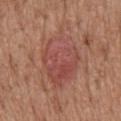Clinical impression: No biopsy was performed on this lesion — it was imaged during a full skin examination and was not determined to be concerning. Clinical summary: The lesion-visualizer software estimated an area of roughly 25 mm², an outline eccentricity of about 0.75 (0 = round, 1 = elongated), and two-axis asymmetry of about 0.15. It also reported a lesion color around L≈49 a*≈26 b*≈26 in CIELAB and a normalized border contrast of about 6. The subject is a male about 60 years old. On the mid back. Imaged with white-light lighting. Approximately 8 mm at its widest. A 15 mm close-up extracted from a 3D total-body photography capture.